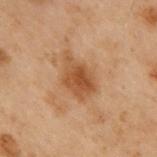Case summary:
• follow-up: catalogued during a skin exam; not biopsied
• body site: the upper back
• tile lighting: cross-polarized
• TBP lesion metrics: an area of roughly 10 mm², an outline eccentricity of about 0.75 (0 = round, 1 = elongated), and two-axis asymmetry of about 0.3; a lesion–skin lightness drop of about 9 and a normalized border contrast of about 7.5; a border-irregularity index near 3/10 and a within-lesion color-variation index near 4/10; a nevus-likeness score of about 65/100 and a detector confidence of about 100 out of 100 that the crop contains a lesion
• image source: ~15 mm tile from a whole-body skin photo
• lesion size: ≈4.5 mm
• subject: male, in their mid- to late 50s